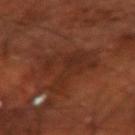A male patient aged 68 to 72. Located on the right forearm. The recorded lesion diameter is about 6 mm. A lesion tile, about 15 mm wide, cut from a 3D total-body photograph.